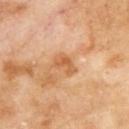Q: Was this lesion biopsied?
A: total-body-photography surveillance lesion; no biopsy
Q: What is the imaging modality?
A: ~15 mm crop, total-body skin-cancer survey
Q: Where on the body is the lesion?
A: the upper back
Q: Who is the patient?
A: male, roughly 70 years of age